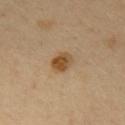* workup · catalogued during a skin exam; not biopsied
* tile lighting · cross-polarized
* image source · total-body-photography crop, ~15 mm field of view
* patient · male, aged approximately 50
* anatomic site · the back